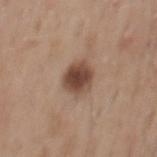site: the mid back
patient: male, aged approximately 55
image source: ~15 mm crop, total-body skin-cancer survey
lighting: white-light illumination
size: ≈3.5 mm